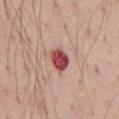Clinical impression: Recorded during total-body skin imaging; not selected for excision or biopsy. Background: An algorithmic analysis of the crop reported an area of roughly 6 mm², an outline eccentricity of about 0.65 (0 = round, 1 = elongated), and a symmetry-axis asymmetry near 0.2. And it measured a lesion color around L≈49 a*≈33 b*≈24 in CIELAB, roughly 18 lightness units darker than nearby skin, and a lesion-to-skin contrast of about 12 (normalized; higher = more distinct). The software also gave a border-irregularity rating of about 1.5/10, a color-variation rating of about 5.5/10, and radial color variation of about 1.5. A male subject in their 40s. A 15 mm close-up tile from a total-body photography series done for melanoma screening. The lesion is on the chest. About 3 mm across. Imaged with white-light lighting.